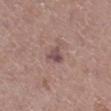Assessment:
Captured during whole-body skin photography for melanoma surveillance; the lesion was not biopsied.
Acquisition and patient details:
Automated image analysis of the tile measured a lesion area of about 3.5 mm². And it measured a border-irregularity rating of about 3.5/10. The software also gave an automated nevus-likeness rating near 0 out of 100. Longest diameter approximately 2.5 mm. On the right lower leg. Cropped from a total-body skin-imaging series; the visible field is about 15 mm. A female patient, aged 48 to 52. Imaged with white-light lighting.